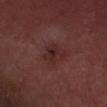Clinical summary: Located on the head or neck. This image is a 15 mm lesion crop taken from a total-body photograph. Longest diameter approximately 3 mm. This is a white-light tile. A male patient, aged approximately 60. Conclusion: Histopathological examination showed a nodular basal cell carcinoma.